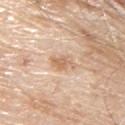Assessment:
Imaged during a routine full-body skin examination; the lesion was not biopsied and no histopathology is available.
Image and clinical context:
Automated image analysis of the tile measured internal color variation of about 2.5 on a 0–10 scale and radial color variation of about 1. It also reported an automated nevus-likeness rating near 0 out of 100. Imaged with white-light lighting. Longest diameter approximately 3 mm. The lesion is located on the upper back. A male subject, about 80 years old. A close-up tile cropped from a whole-body skin photograph, about 15 mm across.